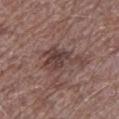Impression:
No biopsy was performed on this lesion — it was imaged during a full skin examination and was not determined to be concerning.
Acquisition and patient details:
The lesion-visualizer software estimated border irregularity of about 7.5 on a 0–10 scale, internal color variation of about 3.5 on a 0–10 scale, and peripheral color asymmetry of about 1. It also reported lesion-presence confidence of about 100/100. The subject is a male aged approximately 70. Located on the left lower leg. A close-up tile cropped from a whole-body skin photograph, about 15 mm across.